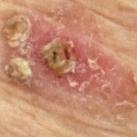Imaged during a routine full-body skin examination; the lesion was not biopsied and no histopathology is available.
The subject is a male roughly 70 years of age.
Cropped from a total-body skin-imaging series; the visible field is about 15 mm.
The lesion is located on the upper back.
This is a cross-polarized tile.